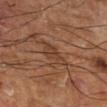Captured during whole-body skin photography for melanoma surveillance; the lesion was not biopsied.
A 15 mm close-up tile from a total-body photography series done for melanoma screening.
A male patient aged approximately 65.
On the right lower leg.